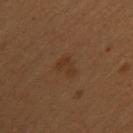The lesion was photographed on a routine skin check and not biopsied; there is no pathology result.
This image is a 15 mm lesion crop taken from a total-body photograph.
The tile uses cross-polarized illumination.
Measured at roughly 3 mm in maximum diameter.
A female subject in their 50s.
From the left upper arm.